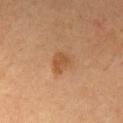Assessment: The lesion was tiled from a total-body skin photograph and was not biopsied. Clinical summary: A male patient in their mid- to late 50s. A 15 mm close-up extracted from a 3D total-body photography capture. Captured under cross-polarized illumination. From the chest. Measured at roughly 3 mm in maximum diameter.